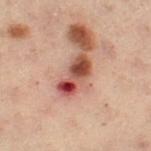Acquisition and patient details:
Captured under cross-polarized illumination. The lesion-visualizer software estimated a lesion area of about 7.5 mm², an eccentricity of roughly 0.95, and two-axis asymmetry of about 0.25. The software also gave roughly 16 lightness units darker than nearby skin and a normalized border contrast of about 12. A female patient approximately 55 years of age. The lesion is located on the left thigh. A close-up tile cropped from a whole-body skin photograph, about 15 mm across.